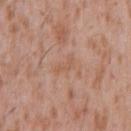Case summary:
• follow-up: catalogued during a skin exam; not biopsied
• imaging modality: ~15 mm tile from a whole-body skin photo
• automated lesion analysis: a lesion area of about 3.5 mm², an eccentricity of roughly 0.9, and two-axis asymmetry of about 0.45; about 5 CIELAB-L* units darker than the surrounding skin and a normalized lesion–skin contrast near 4.5
• illumination: white-light
• patient: male, about 45 years old
• body site: the chest
• diameter: ≈3 mm